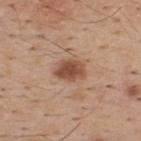lighting: white-light
body site: the back
size: about 3.5 mm
patient: male, aged 38 to 42
acquisition: ~15 mm tile from a whole-body skin photo